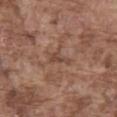Impression: Recorded during total-body skin imaging; not selected for excision or biopsy. Image and clinical context: The subject is a male approximately 75 years of age. Located on the front of the torso. This image is a 15 mm lesion crop taken from a total-body photograph. An algorithmic analysis of the crop reported an eccentricity of roughly 0.9 and a symmetry-axis asymmetry near 0.55. It also reported a lesion–skin lightness drop of about 8 and a lesion-to-skin contrast of about 6 (normalized; higher = more distinct). The analysis additionally found a border-irregularity rating of about 5.5/10, internal color variation of about 0 on a 0–10 scale, and peripheral color asymmetry of about 0. The software also gave an automated nevus-likeness rating near 0 out of 100 and a lesion-detection confidence of about 80/100.